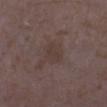Assessment: Captured during whole-body skin photography for melanoma surveillance; the lesion was not biopsied.